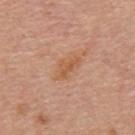follow-up = catalogued during a skin exam; not biopsied | subject = male, aged 53 to 57 | image-analysis metrics = an eccentricity of roughly 0.85 and a symmetry-axis asymmetry near 0.25; a mean CIELAB color near L≈58 a*≈23 b*≈35, a lesion–skin lightness drop of about 7, and a normalized border contrast of about 6; a classifier nevus-likeness of about 0/100 | lesion size = about 4 mm | imaging modality = ~15 mm crop, total-body skin-cancer survey | tile lighting = white-light | anatomic site = the back.